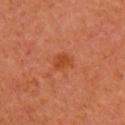Findings:
* workup · no biopsy performed (imaged during a skin exam)
* location · the arm
* patient · female, aged approximately 65
* image source · ~15 mm tile from a whole-body skin photo
* lesion size · ≈2.5 mm